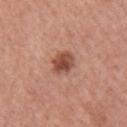Recorded during total-body skin imaging; not selected for excision or biopsy.
The patient is a female roughly 60 years of age.
Imaged with white-light lighting.
On the right upper arm.
A 15 mm crop from a total-body photograph taken for skin-cancer surveillance.